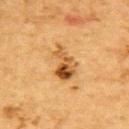Impression:
This lesion was catalogued during total-body skin photography and was not selected for biopsy.
Background:
A male patient, in their mid-80s or older. The lesion's longest dimension is about 4.5 mm. The lesion is located on the upper back. Cropped from a whole-body photographic skin survey; the tile spans about 15 mm.